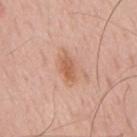notes: imaged on a skin check; not biopsied | patient: male, aged 63–67 | location: the mid back | imaging modality: 15 mm crop, total-body photography.